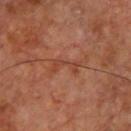Q: Was a biopsy performed?
A: catalogued during a skin exam; not biopsied
Q: What kind of image is this?
A: 15 mm crop, total-body photography
Q: How large is the lesion?
A: about 3.5 mm
Q: What lighting was used for the tile?
A: cross-polarized illumination
Q: Automated lesion metrics?
A: an outline eccentricity of about 0.9 (0 = round, 1 = elongated) and a symmetry-axis asymmetry near 0.55; border irregularity of about 8 on a 0–10 scale and internal color variation of about 0 on a 0–10 scale; a detector confidence of about 95 out of 100 that the crop contains a lesion
Q: What is the anatomic site?
A: the chest
Q: Who is the patient?
A: male, in their mid- to late 60s